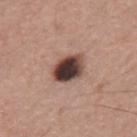Q: Automated lesion metrics?
A: a shape eccentricity near 0.65 and two-axis asymmetry of about 0.2; a lesion color around L≈40 a*≈18 b*≈21 in CIELAB, about 22 CIELAB-L* units darker than the surrounding skin, and a lesion-to-skin contrast of about 16 (normalized; higher = more distinct)
Q: Illumination type?
A: white-light
Q: Patient demographics?
A: male, aged around 55
Q: What is the imaging modality?
A: 15 mm crop, total-body photography